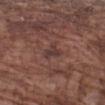Assessment:
Recorded during total-body skin imaging; not selected for excision or biopsy.
Image and clinical context:
The lesion-visualizer software estimated an area of roughly 3.5 mm², an eccentricity of roughly 0.8, and two-axis asymmetry of about 0.45. The recorded lesion diameter is about 2.5 mm. A close-up tile cropped from a whole-body skin photograph, about 15 mm across. From the left forearm. A male patient, in their mid- to late 70s.